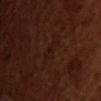Recorded during total-body skin imaging; not selected for excision or biopsy. The lesion-visualizer software estimated a footprint of about 3 mm² and a shape eccentricity near 0.95. The software also gave a mean CIELAB color near L≈16 a*≈20 b*≈21 and a normalized lesion–skin contrast near 4.5. The software also gave a border-irregularity rating of about 5.5/10, a within-lesion color-variation index near 0/10, and a peripheral color-asymmetry measure near 0. Captured under cross-polarized illumination. Located on the chest. The patient is a female approximately 55 years of age. A roughly 15 mm field-of-view crop from a total-body skin photograph. Measured at roughly 3 mm in maximum diameter.